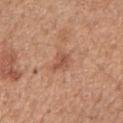Q: How was this image acquired?
A: ~15 mm tile from a whole-body skin photo
Q: Where on the body is the lesion?
A: the right upper arm
Q: Patient demographics?
A: female, roughly 50 years of age
Q: How large is the lesion?
A: ~2.5 mm (longest diameter)